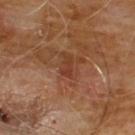follow-up = catalogued during a skin exam; not biopsied
image = total-body-photography crop, ~15 mm field of view
lighting = cross-polarized
site = the chest
lesion diameter = about 2.5 mm
subject = male, in their 60s